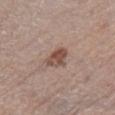<record>
<biopsy_status>not biopsied; imaged during a skin examination</biopsy_status>
<site>right lower leg</site>
<patient>
  <sex>male</sex>
  <age_approx>55</age_approx>
</patient>
<image>
  <source>total-body photography crop</source>
  <field_of_view_mm>15</field_of_view_mm>
</image>
</record>